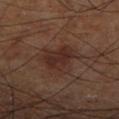No biopsy was performed on this lesion — it was imaged during a full skin examination and was not determined to be concerning.
A close-up tile cropped from a whole-body skin photograph, about 15 mm across.
The lesion is located on the leg.
A male subject aged around 70.
About 5 mm across.
This is a cross-polarized tile.